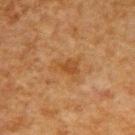follow-up: total-body-photography surveillance lesion; no biopsy | acquisition: ~15 mm crop, total-body skin-cancer survey | location: the upper back | diameter: ~3.5 mm (longest diameter) | patient: male, aged 48–52 | tile lighting: cross-polarized illumination.